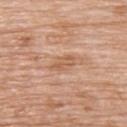Imaged during a routine full-body skin examination; the lesion was not biopsied and no histopathology is available. The lesion is located on the upper back. The tile uses white-light illumination. A female subject about 70 years old. A 15 mm close-up tile from a total-body photography series done for melanoma screening. The lesion's longest dimension is about 3 mm.